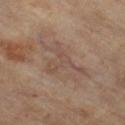Q: Is there a histopathology result?
A: no biopsy performed (imaged during a skin exam)
Q: What are the patient's age and sex?
A: male, roughly 60 years of age
Q: What kind of image is this?
A: 15 mm crop, total-body photography
Q: What is the anatomic site?
A: the right thigh